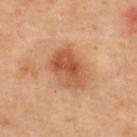Captured during whole-body skin photography for melanoma surveillance; the lesion was not biopsied.
Measured at roughly 5 mm in maximum diameter.
Located on the back.
A female patient about 40 years old.
Imaged with cross-polarized lighting.
A lesion tile, about 15 mm wide, cut from a 3D total-body photograph.